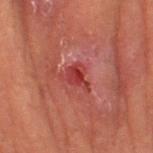  biopsy_status: not biopsied; imaged during a skin examination
  lesion_size:
    long_diameter_mm_approx: 3.5
  lighting: cross-polarized
  site: leg
  patient:
    sex: female
    age_approx: 80
  image:
    source: total-body photography crop
    field_of_view_mm: 15